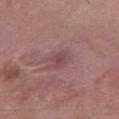The lesion was tiled from a total-body skin photograph and was not biopsied. About 2.5 mm across. The lesion is on the chest. A female patient, about 40 years old. A region of skin cropped from a whole-body photographic capture, roughly 15 mm wide. Imaged with white-light lighting.